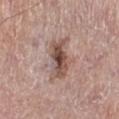The lesion was photographed on a routine skin check and not biopsied; there is no pathology result.
Imaged with white-light lighting.
This image is a 15 mm lesion crop taken from a total-body photograph.
The lesion is on the right lower leg.
The subject is a male aged 73 to 77.
The lesion's longest dimension is about 5 mm.